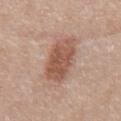Q: Was a biopsy performed?
A: catalogued during a skin exam; not biopsied
Q: What is the lesion's diameter?
A: ≈5.5 mm
Q: What is the imaging modality?
A: 15 mm crop, total-body photography
Q: What is the anatomic site?
A: the mid back
Q: Who is the patient?
A: male, in their mid-50s